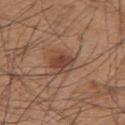Part of a total-body skin-imaging series; this lesion was reviewed on a skin check and was not flagged for biopsy.
Located on the upper back.
An algorithmic analysis of the crop reported a lesion area of about 6.5 mm² and a shape-asymmetry score of about 0.15 (0 = symmetric). It also reported a border-irregularity rating of about 2/10, internal color variation of about 4 on a 0–10 scale, and a peripheral color-asymmetry measure near 1.5.
A 15 mm close-up tile from a total-body photography series done for melanoma screening.
The patient is a male in their mid-40s.
Approximately 3.5 mm at its widest.